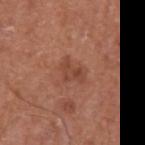No biopsy was performed on this lesion — it was imaged during a full skin examination and was not determined to be concerning.
A male patient, approximately 65 years of age.
Approximately 3 mm at its widest.
The tile uses white-light illumination.
A 15 mm close-up extracted from a 3D total-body photography capture.
From the left upper arm.